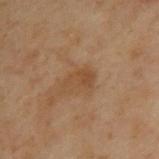Imaged during a routine full-body skin examination; the lesion was not biopsied and no histopathology is available.
A close-up tile cropped from a whole-body skin photograph, about 15 mm across.
An algorithmic analysis of the crop reported an average lesion color of about L≈37 a*≈15 b*≈28 (CIELAB). It also reported border irregularity of about 3.5 on a 0–10 scale and a color-variation rating of about 2/10.
The recorded lesion diameter is about 3.5 mm.
The lesion is located on the upper back.
The tile uses cross-polarized illumination.
A male subject, roughly 55 years of age.